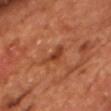Part of a total-body skin-imaging series; this lesion was reviewed on a skin check and was not flagged for biopsy. The total-body-photography lesion software estimated an area of roughly 4.5 mm², a shape eccentricity near 0.9, and two-axis asymmetry of about 0.5. The analysis additionally found a lesion color around L≈41 a*≈28 b*≈35 in CIELAB, a lesion–skin lightness drop of about 9, and a normalized lesion–skin contrast near 7. A male subject aged around 65. A lesion tile, about 15 mm wide, cut from a 3D total-body photograph. The lesion's longest dimension is about 3.5 mm. This is a cross-polarized tile.